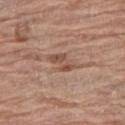This lesion was catalogued during total-body skin photography and was not selected for biopsy. From the right thigh. Cropped from a total-body skin-imaging series; the visible field is about 15 mm. A female subject, approximately 80 years of age. The lesion-visualizer software estimated a color-variation rating of about 3.5/10. The software also gave a nevus-likeness score of about 0/100 and a lesion-detection confidence of about 100/100.